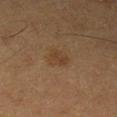Part of a total-body skin-imaging series; this lesion was reviewed on a skin check and was not flagged for biopsy. The lesion's longest dimension is about 2.5 mm. The lesion is located on the left lower leg. A 15 mm crop from a total-body photograph taken for skin-cancer surveillance. This is a cross-polarized tile. A male subject aged 53 to 57.